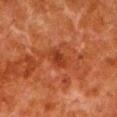workup=imaged on a skin check; not biopsied
image-analysis metrics=a lesion area of about 3.5 mm², an eccentricity of roughly 0.8, and a symmetry-axis asymmetry near 0.25
lighting=cross-polarized illumination
anatomic site=the left lower leg
image source=~15 mm crop, total-body skin-cancer survey
subject=male, roughly 80 years of age